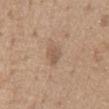{
  "automated_metrics": {
    "cielab_L": 56,
    "cielab_a": 16,
    "cielab_b": 28,
    "vs_skin_darker_L": 8.0,
    "vs_skin_contrast_norm": 6.0,
    "nevus_likeness_0_100": 5,
    "lesion_detection_confidence_0_100": 100
  },
  "patient": {
    "sex": "male",
    "age_approx": 65
  },
  "image": {
    "source": "total-body photography crop",
    "field_of_view_mm": 15
  },
  "site": "chest",
  "lighting": "white-light",
  "lesion_size": {
    "long_diameter_mm_approx": 3.0
  }
}